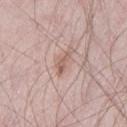notes: no biopsy performed (imaged during a skin exam)
illumination: white-light illumination
acquisition: ~15 mm crop, total-body skin-cancer survey
body site: the leg
size: ≈2.5 mm
automated lesion analysis: an area of roughly 2.5 mm², an eccentricity of roughly 0.95, and a symmetry-axis asymmetry near 0.45; an average lesion color of about L≈58 a*≈20 b*≈24 (CIELAB), roughly 10 lightness units darker than nearby skin, and a lesion-to-skin contrast of about 6.5 (normalized; higher = more distinct)
patient: male, in their mid-20s